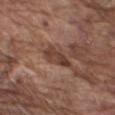Captured during whole-body skin photography for melanoma surveillance; the lesion was not biopsied.
The tile uses white-light illumination.
A male subject aged approximately 75.
Measured at roughly 4 mm in maximum diameter.
On the right upper arm.
A 15 mm close-up extracted from a 3D total-body photography capture.
Automated tile analysis of the lesion measured an average lesion color of about L≈39 a*≈20 b*≈26 (CIELAB), a lesion–skin lightness drop of about 11, and a lesion-to-skin contrast of about 9 (normalized; higher = more distinct).